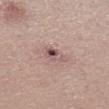Assessment:
The lesion was tiled from a total-body skin photograph and was not biopsied.
Clinical summary:
Cropped from a whole-body photographic skin survey; the tile spans about 15 mm. The patient is a female approximately 20 years of age. The tile uses white-light illumination. Longest diameter approximately 3 mm. On the left lower leg.